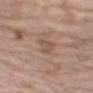{
  "biopsy_status": "not biopsied; imaged during a skin examination",
  "lighting": "white-light",
  "image": {
    "source": "total-body photography crop",
    "field_of_view_mm": 15
  },
  "lesion_size": {
    "long_diameter_mm_approx": 3.5
  },
  "patient": {
    "sex": "male",
    "age_approx": 70
  },
  "site": "right upper arm"
}